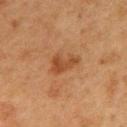This lesion was catalogued during total-body skin photography and was not selected for biopsy. This image is a 15 mm lesion crop taken from a total-body photograph. The patient is a male about 75 years old. The lesion-visualizer software estimated a nevus-likeness score of about 15/100 and a detector confidence of about 100 out of 100 that the crop contains a lesion. The lesion is located on the mid back.